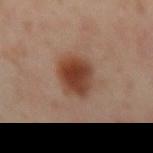Recorded during total-body skin imaging; not selected for excision or biopsy. The subject is a female aged 38–42. Measured at roughly 4 mm in maximum diameter. The tile uses cross-polarized illumination. On the left arm. A roughly 15 mm field-of-view crop from a total-body skin photograph.